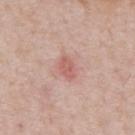notes = imaged on a skin check; not biopsied
body site = the back
acquisition = total-body-photography crop, ~15 mm field of view
subject = male, aged 73–77